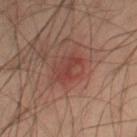Imaged during a routine full-body skin examination; the lesion was not biopsied and no histopathology is available. A male subject aged 53–57. The tile uses cross-polarized illumination. On the right thigh. This image is a 15 mm lesion crop taken from a total-body photograph. Automated tile analysis of the lesion measured a classifier nevus-likeness of about 0/100 and lesion-presence confidence of about 100/100. The lesion's longest dimension is about 4 mm.